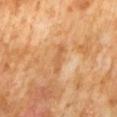The patient is a male in their 60s. The lesion's longest dimension is about 3.5 mm. Cropped from a whole-body photographic skin survey; the tile spans about 15 mm. The lesion-visualizer software estimated a footprint of about 3.5 mm² and a shape eccentricity near 0.95. Located on the abdomen.